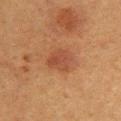Case summary:
* follow-up: no biopsy performed (imaged during a skin exam)
* tile lighting: cross-polarized illumination
* acquisition: 15 mm crop, total-body photography
* image-analysis metrics: a lesion area of about 7 mm², an eccentricity of roughly 0.6, and a symmetry-axis asymmetry near 0.25; border irregularity of about 2.5 on a 0–10 scale and internal color variation of about 3 on a 0–10 scale; a classifier nevus-likeness of about 70/100 and a detector confidence of about 100 out of 100 that the crop contains a lesion
* subject: female, aged around 40
* location: the upper back
* size: ~3.5 mm (longest diameter)